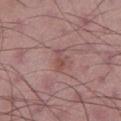{
  "biopsy_status": "not biopsied; imaged during a skin examination",
  "lesion_size": {
    "long_diameter_mm_approx": 3.0
  },
  "site": "left thigh",
  "patient": {
    "sex": "male",
    "age_approx": 50
  },
  "image": {
    "source": "total-body photography crop",
    "field_of_view_mm": 15
  },
  "lighting": "white-light"
}